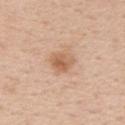The lesion was photographed on a routine skin check and not biopsied; there is no pathology result.
About 3 mm across.
A male subject approximately 55 years of age.
From the chest.
The tile uses white-light illumination.
Automated image analysis of the tile measured a border-irregularity index near 1.5/10. And it measured a nevus-likeness score of about 55/100 and a lesion-detection confidence of about 100/100.
A 15 mm close-up extracted from a 3D total-body photography capture.